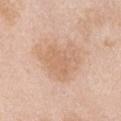Clinical impression:
Recorded during total-body skin imaging; not selected for excision or biopsy.
Context:
A female subject, aged 63–67. The total-body-photography lesion software estimated a shape eccentricity near 0.4 and a shape-asymmetry score of about 0.35 (0 = symmetric). The analysis additionally found about 7 CIELAB-L* units darker than the surrounding skin and a normalized lesion–skin contrast near 5. A region of skin cropped from a whole-body photographic capture, roughly 15 mm wide. The lesion's longest dimension is about 5.5 mm. The tile uses white-light illumination. The lesion is on the front of the torso.